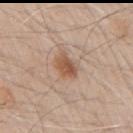Q: Was a biopsy performed?
A: no biopsy performed (imaged during a skin exam)
Q: Who is the patient?
A: male, aged 68 to 72
Q: Where on the body is the lesion?
A: the right upper arm
Q: What kind of image is this?
A: 15 mm crop, total-body photography
Q: What is the lesion's diameter?
A: ≈3.5 mm